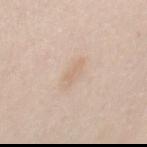follow-up = imaged on a skin check; not biopsied
lesion size = ~3 mm (longest diameter)
lighting = white-light
subject = female, aged 63 to 67
acquisition = 15 mm crop, total-body photography
body site = the chest